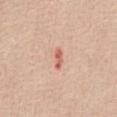Cropped from a total-body skin-imaging series; the visible field is about 15 mm.
Approximately 2.5 mm at its widest.
A female patient in their mid- to late 50s.
Automated tile analysis of the lesion measured a lesion area of about 2.5 mm², an eccentricity of roughly 0.9, and a symmetry-axis asymmetry near 0.3. And it measured internal color variation of about 0 on a 0–10 scale and peripheral color asymmetry of about 0.
On the front of the torso.
This is a white-light tile.